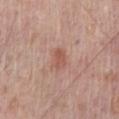biopsy status: catalogued during a skin exam; not biopsied | subject: male, roughly 70 years of age | automated lesion analysis: a lesion area of about 4 mm², an outline eccentricity of about 0.75 (0 = round, 1 = elongated), and a symmetry-axis asymmetry near 0.25; border irregularity of about 2 on a 0–10 scale, internal color variation of about 2.5 on a 0–10 scale, and peripheral color asymmetry of about 1 | size: ≈2.5 mm | imaging modality: ~15 mm crop, total-body skin-cancer survey | illumination: white-light | anatomic site: the chest.